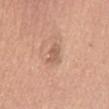<record>
<biopsy_status>not biopsied; imaged during a skin examination</biopsy_status>
<lesion_size>
  <long_diameter_mm_approx>2.5</long_diameter_mm_approx>
</lesion_size>
<patient>
  <sex>female</sex>
  <age_approx>55</age_approx>
</patient>
<site>mid back</site>
<image>
  <source>total-body photography crop</source>
  <field_of_view_mm>15</field_of_view_mm>
</image>
<lighting>white-light</lighting>
</record>